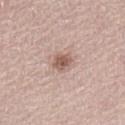Part of a total-body skin-imaging series; this lesion was reviewed on a skin check and was not flagged for biopsy.
This image is a 15 mm lesion crop taken from a total-body photograph.
Measured at roughly 2.5 mm in maximum diameter.
A female subject, aged 63–67.
From the abdomen.